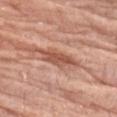| key | value |
|---|---|
| biopsy status | imaged on a skin check; not biopsied |
| lesion size | ~5 mm (longest diameter) |
| TBP lesion metrics | an area of roughly 7.5 mm², a shape eccentricity near 0.9, and two-axis asymmetry of about 0.3; a mean CIELAB color near L≈54 a*≈25 b*≈30, roughly 12 lightness units darker than nearby skin, and a lesion-to-skin contrast of about 8 (normalized; higher = more distinct); radial color variation of about 1; an automated nevus-likeness rating near 0 out of 100 and a lesion-detection confidence of about 85/100 |
| patient | female, in their mid- to late 70s |
| anatomic site | the left forearm |
| illumination | white-light |
| image source | total-body-photography crop, ~15 mm field of view |